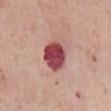Part of a total-body skin-imaging series; this lesion was reviewed on a skin check and was not flagged for biopsy. The subject is a female in their mid-60s. Located on the chest. A 15 mm close-up extracted from a 3D total-body photography capture.